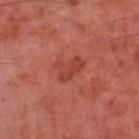Impression:
Part of a total-body skin-imaging series; this lesion was reviewed on a skin check and was not flagged for biopsy.
Background:
The lesion is located on the left thigh. Automated image analysis of the tile measured a border-irregularity rating of about 4.5/10 and internal color variation of about 2.5 on a 0–10 scale. A region of skin cropped from a whole-body photographic capture, roughly 15 mm wide. The subject is a male in their 70s. Captured under cross-polarized illumination.